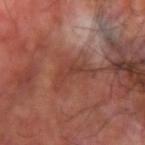Recorded during total-body skin imaging; not selected for excision or biopsy.
Imaged with cross-polarized lighting.
A male subject, aged approximately 60.
The lesion's longest dimension is about 5 mm.
This image is a 15 mm lesion crop taken from a total-body photograph.
The lesion is on the left forearm.